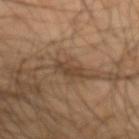On the left forearm. Cropped from a total-body skin-imaging series; the visible field is about 15 mm. The lesion-visualizer software estimated an area of roughly 4.5 mm² and a shape eccentricity near 0.9. Measured at roughly 3.5 mm in maximum diameter. The subject is a male approximately 50 years of age. Imaged with cross-polarized lighting.